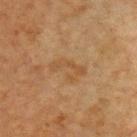<record>
  <lighting>cross-polarized</lighting>
  <patient>
    <sex>male</sex>
    <age_approx>65</age_approx>
  </patient>
  <lesion_size>
    <long_diameter_mm_approx>4.0</long_diameter_mm_approx>
  </lesion_size>
  <site>chest</site>
  <image>
    <source>total-body photography crop</source>
    <field_of_view_mm>15</field_of_view_mm>
  </image>
</record>